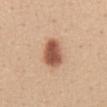Notes:
* biopsy status — catalogued during a skin exam; not biopsied
* patient — female, in their mid-40s
* site — the abdomen
* acquisition — 15 mm crop, total-body photography
* lesion diameter — about 4 mm
* illumination — white-light illumination